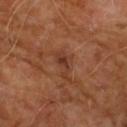Clinical impression:
No biopsy was performed on this lesion — it was imaged during a full skin examination and was not determined to be concerning.
Acquisition and patient details:
Cropped from a whole-body photographic skin survey; the tile spans about 15 mm. Measured at roughly 3.5 mm in maximum diameter. On the front of the torso. The tile uses cross-polarized illumination. The patient is a male aged 58 to 62.